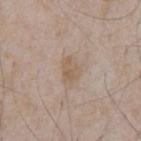Imaged during a routine full-body skin examination; the lesion was not biopsied and no histopathology is available. The lesion is on the chest. The recorded lesion diameter is about 3 mm. Captured under white-light illumination. A male subject aged 63 to 67. Cropped from a whole-body photographic skin survey; the tile spans about 15 mm.